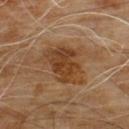Findings:
- workup · total-body-photography surveillance lesion; no biopsy
- acquisition · ~15 mm crop, total-body skin-cancer survey
- patient · male, about 60 years old
- diameter · ~6 mm (longest diameter)
- body site · the chest
- tile lighting · cross-polarized
- TBP lesion metrics · a footprint of about 17 mm² and an outline eccentricity of about 0.75 (0 = round, 1 = elongated); a lesion color around L≈39 a*≈19 b*≈33 in CIELAB, a lesion–skin lightness drop of about 9, and a normalized lesion–skin contrast near 8